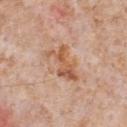Acquisition and patient details: Imaged with white-light lighting. The lesion is located on the chest. The lesion's longest dimension is about 5 mm. A 15 mm close-up tile from a total-body photography series done for melanoma screening. A male patient in their mid- to late 60s.